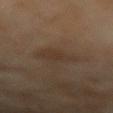<lesion>
<biopsy_status>not biopsied; imaged during a skin examination</biopsy_status>
<patient>
  <sex>female</sex>
  <age_approx>60</age_approx>
</patient>
<automated_metrics>
  <cielab_L>31</cielab_L>
  <cielab_a>12</cielab_a>
  <cielab_b>23</cielab_b>
  <vs_skin_darker_L>5.0</vs_skin_darker_L>
  <color_variation_0_10>1.5</color_variation_0_10>
  <peripheral_color_asymmetry>0.5</peripheral_color_asymmetry>
</automated_metrics>
<image>
  <source>total-body photography crop</source>
  <field_of_view_mm>15</field_of_view_mm>
</image>
<lesion_size>
  <long_diameter_mm_approx>3.0</long_diameter_mm_approx>
</lesion_size>
<site>left forearm</site>
</lesion>